On the mid back · a male subject, aged 58 to 62 · a 15 mm close-up tile from a total-body photography series done for melanoma screening: 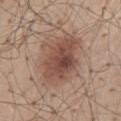diagnosis = a dysplastic (Clark) nevus